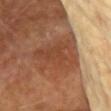Part of a total-body skin-imaging series; this lesion was reviewed on a skin check and was not flagged for biopsy. Automated tile analysis of the lesion measured a lesion color around L≈45 a*≈24 b*≈33 in CIELAB and a lesion-to-skin contrast of about 6.5 (normalized; higher = more distinct). The software also gave a border-irregularity index near 5/10 and a peripheral color-asymmetry measure near 1. Measured at roughly 5.5 mm in maximum diameter. A female subject, aged around 75. Imaged with cross-polarized lighting. The lesion is located on the head or neck. A region of skin cropped from a whole-body photographic capture, roughly 15 mm wide.